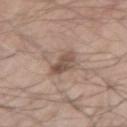Q: Was this lesion biopsied?
A: total-body-photography surveillance lesion; no biopsy
Q: What lighting was used for the tile?
A: white-light
Q: What is the lesion's diameter?
A: about 3 mm
Q: Lesion location?
A: the arm
Q: What is the imaging modality?
A: total-body-photography crop, ~15 mm field of view
Q: Automated lesion metrics?
A: an eccentricity of roughly 0.5 and two-axis asymmetry of about 0.3; internal color variation of about 4 on a 0–10 scale and radial color variation of about 1.5; an automated nevus-likeness rating near 25 out of 100 and a detector confidence of about 100 out of 100 that the crop contains a lesion
Q: Who is the patient?
A: male, roughly 70 years of age